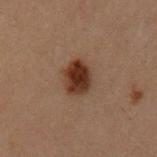biopsy status: catalogued during a skin exam; not biopsied
subject: female, roughly 30 years of age
anatomic site: the arm
image-analysis metrics: an outline eccentricity of about 0.7 (0 = round, 1 = elongated) and a symmetry-axis asymmetry near 0.15; a mean CIELAB color near L≈25 a*≈18 b*≈23 and a lesion-to-skin contrast of about 13 (normalized; higher = more distinct); lesion-presence confidence of about 100/100
image source: ~15 mm crop, total-body skin-cancer survey
illumination: cross-polarized illumination